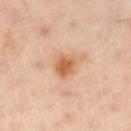workup = catalogued during a skin exam; not biopsied
location = the left leg
tile lighting = cross-polarized illumination
imaging modality = ~15 mm tile from a whole-body skin photo
patient = female, aged 38 to 42
size = ≈3 mm